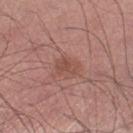Case summary:
– workup · no biopsy performed (imaged during a skin exam)
– patient · male, aged approximately 70
– location · the right thigh
– illumination · white-light illumination
– size · ≈2.5 mm
– image source · 15 mm crop, total-body photography
– image-analysis metrics · a footprint of about 4.5 mm² and an eccentricity of roughly 0.65; a lesion color around L≈49 a*≈22 b*≈25 in CIELAB, about 7 CIELAB-L* units darker than the surrounding skin, and a normalized border contrast of about 5.5; a border-irregularity index near 3/10, a within-lesion color-variation index near 2.5/10, and peripheral color asymmetry of about 1; a nevus-likeness score of about 0/100 and a lesion-detection confidence of about 100/100